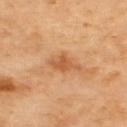notes — imaged on a skin check; not biopsied | tile lighting — cross-polarized illumination | size — ~3.5 mm (longest diameter) | location — the upper back | image source — 15 mm crop, total-body photography | TBP lesion metrics — a mean CIELAB color near L≈58 a*≈26 b*≈42, about 10 CIELAB-L* units darker than the surrounding skin, and a lesion-to-skin contrast of about 6.5 (normalized; higher = more distinct).